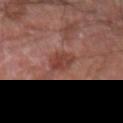This lesion was catalogued during total-body skin photography and was not selected for biopsy. The lesion's longest dimension is about 3 mm. An algorithmic analysis of the crop reported a footprint of about 6 mm², an outline eccentricity of about 0.65 (0 = round, 1 = elongated), and two-axis asymmetry of about 0.2. It also reported about 8 CIELAB-L* units darker than the surrounding skin. The lesion is located on the arm. Cropped from a whole-body photographic skin survey; the tile spans about 15 mm. A male patient, approximately 60 years of age.